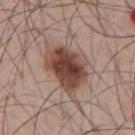Q: Was a biopsy performed?
A: catalogued during a skin exam; not biopsied
Q: What did automated image analysis measure?
A: an area of roughly 19 mm², an eccentricity of roughly 0.8, and a symmetry-axis asymmetry near 0.25
Q: Who is the patient?
A: male, aged around 55
Q: Illumination type?
A: white-light
Q: What kind of image is this?
A: ~15 mm crop, total-body skin-cancer survey
Q: What is the anatomic site?
A: the upper back